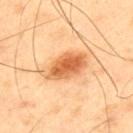The lesion was tiled from a total-body skin photograph and was not biopsied.